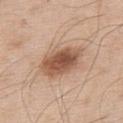Assessment: Recorded during total-body skin imaging; not selected for excision or biopsy. Context: The lesion is located on the upper back. An algorithmic analysis of the crop reported a shape eccentricity near 0.7 and two-axis asymmetry of about 0.15. The analysis additionally found a lesion color around L≈54 a*≈21 b*≈30 in CIELAB, roughly 14 lightness units darker than nearby skin, and a normalized lesion–skin contrast near 9.5. The analysis additionally found internal color variation of about 5.5 on a 0–10 scale and peripheral color asymmetry of about 1.5. It also reported a detector confidence of about 100 out of 100 that the crop contains a lesion. A 15 mm crop from a total-body photograph taken for skin-cancer surveillance. Approximately 5.5 mm at its widest. This is a white-light tile. A male subject, aged around 55.